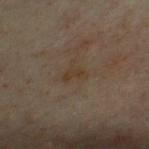acquisition: total-body-photography crop, ~15 mm field of view | subject: male, aged around 50 | anatomic site: the front of the torso.